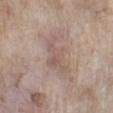{"patient": {"sex": "male", "age_approx": 60}, "lesion_size": {"long_diameter_mm_approx": 5.0}, "site": "left lower leg", "lighting": "white-light", "image": {"source": "total-body photography crop", "field_of_view_mm": 15}}